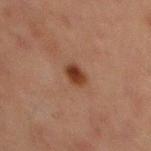workup = no biopsy performed (imaged during a skin exam) | acquisition = ~15 mm tile from a whole-body skin photo | location = the abdomen | patient = male, aged 63 to 67.